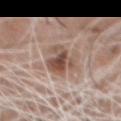workup = total-body-photography surveillance lesion; no biopsy
image source = ~15 mm crop, total-body skin-cancer survey
body site = the mid back
size = about 5 mm
tile lighting = white-light
patient = male, approximately 60 years of age